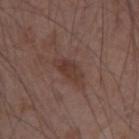notes = no biopsy performed (imaged during a skin exam) | subject = male, aged 48–52 | size = about 3.5 mm | image-analysis metrics = a shape eccentricity near 0.85 and two-axis asymmetry of about 0.25; a lesion color around L≈36 a*≈18 b*≈23 in CIELAB, a lesion–skin lightness drop of about 7, and a normalized border contrast of about 6.5; a border-irregularity index near 2.5/10, a within-lesion color-variation index near 2.5/10, and peripheral color asymmetry of about 1 | image = total-body-photography crop, ~15 mm field of view | illumination = white-light illumination | location = the left upper arm.